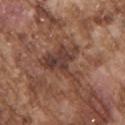{
  "biopsy_status": "not biopsied; imaged during a skin examination",
  "site": "chest",
  "patient": {
    "sex": "male",
    "age_approx": 75
  },
  "image": {
    "source": "total-body photography crop",
    "field_of_view_mm": 15
  }
}